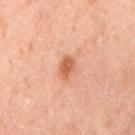Captured during whole-body skin photography for melanoma surveillance; the lesion was not biopsied. The lesion is on the mid back. The tile uses cross-polarized illumination. A male subject, aged around 70. A 15 mm close-up extracted from a 3D total-body photography capture.